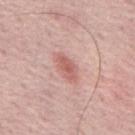Findings:
• biopsy status — catalogued during a skin exam; not biopsied
• location — the mid back
• lighting — white-light illumination
• subject — male, about 65 years old
• diameter — about 3.5 mm
• imaging modality — 15 mm crop, total-body photography